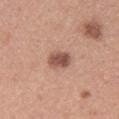Image and clinical context: A female subject, aged approximately 30. Captured under white-light illumination. Automated tile analysis of the lesion measured an automated nevus-likeness rating near 80 out of 100 and a lesion-detection confidence of about 100/100. On the upper back. A region of skin cropped from a whole-body photographic capture, roughly 15 mm wide.